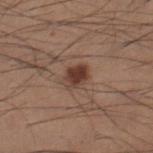Assessment: Captured during whole-body skin photography for melanoma surveillance; the lesion was not biopsied. Image and clinical context: A male subject, aged around 35. Captured under white-light illumination. A roughly 15 mm field-of-view crop from a total-body skin photograph. The lesion is on the right lower leg.